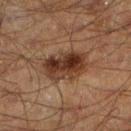* notes: imaged on a skin check; not biopsied
* imaging modality: ~15 mm tile from a whole-body skin photo
* patient: male, about 50 years old
* location: the left lower leg
* tile lighting: cross-polarized
* image-analysis metrics: a symmetry-axis asymmetry near 0.15; a border-irregularity rating of about 2/10, a color-variation rating of about 7/10, and peripheral color asymmetry of about 2.5; a lesion-detection confidence of about 100/100